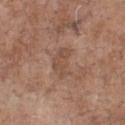Recorded during total-body skin imaging; not selected for excision or biopsy. This image is a 15 mm lesion crop taken from a total-body photograph. From the front of the torso. Imaged with white-light lighting. The recorded lesion diameter is about 4 mm. A male subject roughly 70 years of age.